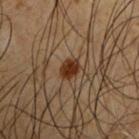follow-up: total-body-photography surveillance lesion; no biopsy | location: the left upper arm | TBP lesion metrics: a mean CIELAB color near L≈23 a*≈16 b*≈24, roughly 11 lightness units darker than nearby skin, and a normalized lesion–skin contrast near 12.5; a border-irregularity index near 2/10; an automated nevus-likeness rating near 95 out of 100 and a detector confidence of about 100 out of 100 that the crop contains a lesion | patient: male, roughly 50 years of age | image: ~15 mm crop, total-body skin-cancer survey | illumination: cross-polarized | diameter: about 2.5 mm.